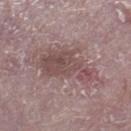Notes:
* workup: imaged on a skin check; not biopsied
* lesion size: ≈6.5 mm
* patient: male, approximately 75 years of age
* acquisition: 15 mm crop, total-body photography
* automated lesion analysis: a mean CIELAB color near L≈48 a*≈19 b*≈18, about 9 CIELAB-L* units darker than the surrounding skin, and a normalized border contrast of about 7; border irregularity of about 6.5 on a 0–10 scale and peripheral color asymmetry of about 1.5
* location: the right lower leg
* illumination: white-light illumination